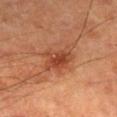Captured during whole-body skin photography for melanoma surveillance; the lesion was not biopsied. A 15 mm close-up extracted from a 3D total-body photography capture. A male subject aged 63 to 67. On the leg. This is a cross-polarized tile.